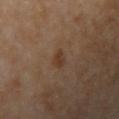This lesion was catalogued during total-body skin photography and was not selected for biopsy.
The lesion is located on the right upper arm.
Cropped from a whole-body photographic skin survey; the tile spans about 15 mm.
A female subject, roughly 65 years of age.